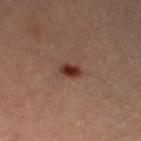Recorded during total-body skin imaging; not selected for excision or biopsy.